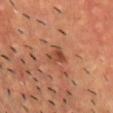<record>
  <biopsy_status>not biopsied; imaged during a skin examination</biopsy_status>
  <site>chest</site>
  <automated_metrics>
    <nevus_likeness_0_100>35</nevus_likeness_0_100>
  </automated_metrics>
  <image>
    <source>total-body photography crop</source>
    <field_of_view_mm>15</field_of_view_mm>
  </image>
  <lighting>cross-polarized</lighting>
  <lesion_size>
    <long_diameter_mm_approx>2.5</long_diameter_mm_approx>
  </lesion_size>
  <patient>
    <sex>male</sex>
    <age_approx>55</age_approx>
  </patient>
</record>